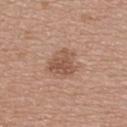No biopsy was performed on this lesion — it was imaged during a full skin examination and was not determined to be concerning.
A 15 mm close-up tile from a total-body photography series done for melanoma screening.
Captured under white-light illumination.
The lesion is located on the back.
Approximately 4 mm at its widest.
The patient is a female aged around 60.